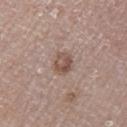The lesion was tiled from a total-body skin photograph and was not biopsied.
A lesion tile, about 15 mm wide, cut from a 3D total-body photograph.
From the leg.
The patient is a male in their 80s.
The tile uses white-light illumination.
Approximately 2.5 mm at its widest.